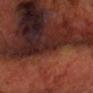Notes:
• illumination: cross-polarized illumination
• diameter: ≈1 mm
• image-analysis metrics: a footprint of about 1 mm², an outline eccentricity of about 0.75 (0 = round, 1 = elongated), and two-axis asymmetry of about 0.4; a lesion color around L≈22 a*≈20 b*≈19 in CIELAB, about 4 CIELAB-L* units darker than the surrounding skin, and a lesion-to-skin contrast of about 5 (normalized; higher = more distinct); a nevus-likeness score of about 0/100 and a detector confidence of about 0 out of 100 that the crop contains a lesion
• subject: male, roughly 70 years of age
• acquisition: ~15 mm crop, total-body skin-cancer survey
• site: the head or neck
• histopathologic diagnosis: a solar lentigo (benign)